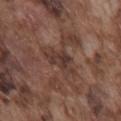No biopsy was performed on this lesion — it was imaged during a full skin examination and was not determined to be concerning. From the chest. Captured under white-light illumination. A male patient, in their mid-70s. This image is a 15 mm lesion crop taken from a total-body photograph. The total-body-photography lesion software estimated an area of roughly 8.5 mm² and a shape eccentricity near 0.9. The software also gave an average lesion color of about L≈36 a*≈17 b*≈22 (CIELAB), about 8 CIELAB-L* units darker than the surrounding skin, and a normalized lesion–skin contrast near 7. And it measured border irregularity of about 4 on a 0–10 scale, internal color variation of about 4 on a 0–10 scale, and peripheral color asymmetry of about 1.